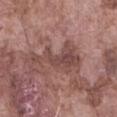{"biopsy_status": "not biopsied; imaged during a skin examination", "site": "abdomen", "image": {"source": "total-body photography crop", "field_of_view_mm": 15}, "patient": {"sex": "male", "age_approx": 75}}